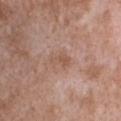Assessment:
Recorded during total-body skin imaging; not selected for excision or biopsy.
Background:
A lesion tile, about 15 mm wide, cut from a 3D total-body photograph. From the chest. A male patient, aged approximately 50. The recorded lesion diameter is about 2.5 mm. The tile uses white-light illumination.